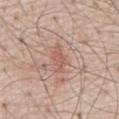The lesion was photographed on a routine skin check and not biopsied; there is no pathology result. On the front of the torso. The subject is a male in their 70s. Cropped from a total-body skin-imaging series; the visible field is about 15 mm. Approximately 3.5 mm at its widest.